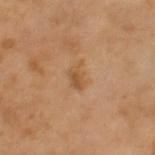The patient is a male aged 63–67.
Captured under cross-polarized illumination.
The lesion's longest dimension is about 3 mm.
This image is a 15 mm lesion crop taken from a total-body photograph.